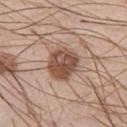notes: catalogued during a skin exam; not biopsied
subject: male, aged 38–42
imaging modality: 15 mm crop, total-body photography
anatomic site: the chest